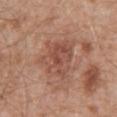Q: Was this lesion biopsied?
A: catalogued during a skin exam; not biopsied
Q: What is the imaging modality?
A: total-body-photography crop, ~15 mm field of view
Q: What are the patient's age and sex?
A: male, approximately 60 years of age
Q: What lighting was used for the tile?
A: white-light
Q: Where on the body is the lesion?
A: the mid back
Q: What is the lesion's diameter?
A: ≈5 mm
Q: Automated lesion metrics?
A: an area of roughly 16 mm² and a shape-asymmetry score of about 0.3 (0 = symmetric); a border-irregularity rating of about 3.5/10 and a color-variation rating of about 4.5/10; an automated nevus-likeness rating near 0 out of 100 and a lesion-detection confidence of about 100/100